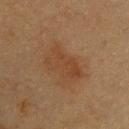  biopsy_status: not biopsied; imaged during a skin examination
  lesion_size:
    long_diameter_mm_approx: 5.0
  image:
    source: total-body photography crop
    field_of_view_mm: 15
  site: upper back
  patient:
    sex: female
    age_approx: 40
  lighting: cross-polarized